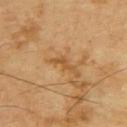Context:
A 15 mm close-up extracted from a 3D total-body photography capture. A male subject, about 70 years old. The lesion is on the upper back. Measured at roughly 3 mm in maximum diameter.